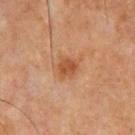Assessment:
Part of a total-body skin-imaging series; this lesion was reviewed on a skin check and was not flagged for biopsy.
Background:
A lesion tile, about 15 mm wide, cut from a 3D total-body photograph. Captured under cross-polarized illumination. From the chest. Approximately 2.5 mm at its widest. A male patient, about 65 years old.